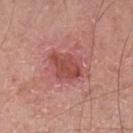Notes:
– follow-up — total-body-photography surveillance lesion; no biopsy
– patient — male, aged around 55
– automated lesion analysis — an average lesion color of about L≈49 a*≈29 b*≈26 (CIELAB), roughly 10 lightness units darker than nearby skin, and a normalized lesion–skin contrast near 7; a classifier nevus-likeness of about 0/100 and lesion-presence confidence of about 100/100
– location — the leg
– imaging modality — ~15 mm tile from a whole-body skin photo